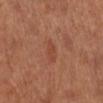notes — no biopsy performed (imaged during a skin exam) | site — the left lower leg | image source — 15 mm crop, total-body photography | tile lighting — white-light illumination | patient — female, aged 63–67 | size — about 2.5 mm.